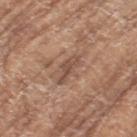Recorded during total-body skin imaging; not selected for excision or biopsy.
A 15 mm close-up tile from a total-body photography series done for melanoma screening.
The subject is a female approximately 75 years of age.
Located on the left thigh.
Imaged with white-light lighting.
The recorded lesion diameter is about 4 mm.